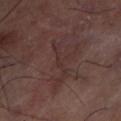Assessment:
This lesion was catalogued during total-body skin photography and was not selected for biopsy.
Acquisition and patient details:
A 15 mm close-up tile from a total-body photography series done for melanoma screening. On the leg. Automated tile analysis of the lesion measured an outline eccentricity of about 0.95 (0 = round, 1 = elongated) and a shape-asymmetry score of about 0.7 (0 = symmetric). The software also gave a mean CIELAB color near L≈31 a*≈17 b*≈17, roughly 5 lightness units darker than nearby skin, and a normalized border contrast of about 5. It also reported border irregularity of about 10 on a 0–10 scale and peripheral color asymmetry of about 0. The software also gave a classifier nevus-likeness of about 0/100 and a detector confidence of about 50 out of 100 that the crop contains a lesion. Captured under cross-polarized illumination.